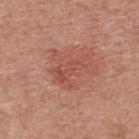The lesion was photographed on a routine skin check and not biopsied; there is no pathology result. A male patient aged approximately 80. The total-body-photography lesion software estimated a mean CIELAB color near L≈51 a*≈27 b*≈28 and a lesion–skin lightness drop of about 7. The analysis additionally found border irregularity of about 6 on a 0–10 scale, a within-lesion color-variation index near 3/10, and radial color variation of about 1. A region of skin cropped from a whole-body photographic capture, roughly 15 mm wide. The lesion is on the back.